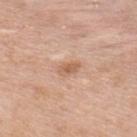Captured during whole-body skin photography for melanoma surveillance; the lesion was not biopsied. Imaged with white-light lighting. A male patient, about 50 years old. About 2.5 mm across. Cropped from a total-body skin-imaging series; the visible field is about 15 mm. On the upper back.